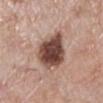Image and clinical context: The total-body-photography lesion software estimated a classifier nevus-likeness of about 85/100. A roughly 15 mm field-of-view crop from a total-body skin photograph. This is a white-light tile. On the left lower leg. Measured at roughly 5.5 mm in maximum diameter. A female subject, approximately 70 years of age. Diagnosis: On excision, pathology confirmed a dysplastic (Clark) nevus.